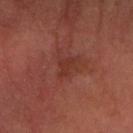This lesion was catalogued during total-body skin photography and was not selected for biopsy. The subject is a male about 65 years old. A close-up tile cropped from a whole-body skin photograph, about 15 mm across. The lesion is located on the left forearm. The lesion-visualizer software estimated an automated nevus-likeness rating near 0 out of 100 and a detector confidence of about 100 out of 100 that the crop contains a lesion.